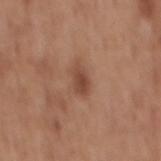follow-up: no biopsy performed (imaged during a skin exam)
image-analysis metrics: a mean CIELAB color near L≈46 a*≈22 b*≈29, a lesion–skin lightness drop of about 10, and a normalized lesion–skin contrast near 7; a classifier nevus-likeness of about 55/100 and lesion-presence confidence of about 100/100
image: 15 mm crop, total-body photography
body site: the mid back
illumination: white-light illumination
subject: male, about 60 years old
lesion size: ~3.5 mm (longest diameter)